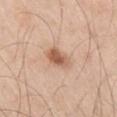biopsy_status: not biopsied; imaged during a skin examination
lesion_size:
  long_diameter_mm_approx: 3.5
patient:
  sex: male
  age_approx: 60
lighting: white-light
image:
  source: total-body photography crop
  field_of_view_mm: 15
site: right thigh
automated_metrics:
  area_mm2_approx: 6.0
  shape_asymmetry: 0.2
  vs_skin_darker_L: 13.0